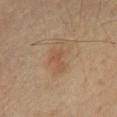image:
  source: total-body photography crop
  field_of_view_mm: 15
lighting: cross-polarized
lesion_size:
  long_diameter_mm_approx: 3.0
site: chest
patient:
  sex: male
  age_approx: 60
automated_metrics:
  area_mm2_approx: 3.5
  eccentricity: 0.85
  shape_asymmetry: 0.5
  nevus_likeness_0_100: 0
  lesion_detection_confidence_0_100: 100